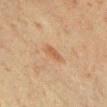Part of a total-body skin-imaging series; this lesion was reviewed on a skin check and was not flagged for biopsy.
The patient is a female about 40 years old.
Approximately 3 mm at its widest.
Imaged with cross-polarized lighting.
From the front of the torso.
A roughly 15 mm field-of-view crop from a total-body skin photograph.
Automated image analysis of the tile measured a shape eccentricity near 0.9 and a symmetry-axis asymmetry near 0.3. It also reported a mean CIELAB color near L≈49 a*≈19 b*≈32 and a lesion-to-skin contrast of about 6 (normalized; higher = more distinct). It also reported a nevus-likeness score of about 55/100 and a detector confidence of about 100 out of 100 that the crop contains a lesion.